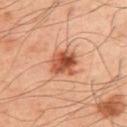Captured during whole-body skin photography for melanoma surveillance; the lesion was not biopsied. This image is a 15 mm lesion crop taken from a total-body photograph. Imaged with cross-polarized lighting. The recorded lesion diameter is about 3.5 mm. A male patient about 50 years old. From the upper back.